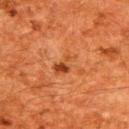Notes:
• imaging modality · ~15 mm crop, total-body skin-cancer survey
• site · the upper back
• patient · male, aged 58 to 62
• lighting · cross-polarized illumination